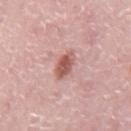- workup: total-body-photography surveillance lesion; no biopsy
- subject: male, in their mid- to late 70s
- image: total-body-photography crop, ~15 mm field of view
- body site: the mid back
- tile lighting: white-light
- size: ≈3 mm